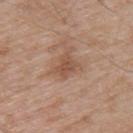Assessment:
Recorded during total-body skin imaging; not selected for excision or biopsy.
Context:
Captured under white-light illumination. The lesion is on the upper back. An algorithmic analysis of the crop reported an average lesion color of about L≈51 a*≈20 b*≈30 (CIELAB), a lesion–skin lightness drop of about 9, and a normalized lesion–skin contrast near 6.5. A 15 mm close-up extracted from a 3D total-body photography capture. The lesion's longest dimension is about 2.5 mm. A male patient in their mid-50s.